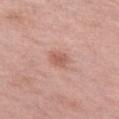A female patient, in their 70s.
The tile uses white-light illumination.
The lesion is located on the left thigh.
The lesion's longest dimension is about 2.5 mm.
A 15 mm crop from a total-body photograph taken for skin-cancer surveillance.